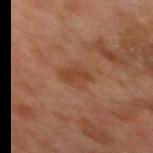No biopsy was performed on this lesion — it was imaged during a full skin examination and was not determined to be concerning.